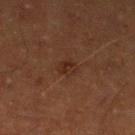biopsy status: imaged on a skin check; not biopsied
patient: male, aged 63–67
image source: 15 mm crop, total-body photography
lesion diameter: ~2.5 mm (longest diameter)
body site: the leg
tile lighting: cross-polarized illumination